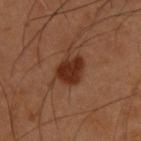Impression: Captured during whole-body skin photography for melanoma surveillance; the lesion was not biopsied. Clinical summary: Approximately 3.5 mm at its widest. Imaged with cross-polarized lighting. A male subject, aged approximately 55. Automated image analysis of the tile measured a mean CIELAB color near L≈29 a*≈23 b*≈29, about 12 CIELAB-L* units darker than the surrounding skin, and a lesion-to-skin contrast of about 11 (normalized; higher = more distinct). The software also gave border irregularity of about 2.5 on a 0–10 scale, a within-lesion color-variation index near 2/10, and radial color variation of about 0.5. It also reported a classifier nevus-likeness of about 100/100 and lesion-presence confidence of about 100/100. A close-up tile cropped from a whole-body skin photograph, about 15 mm across. The lesion is on the back.